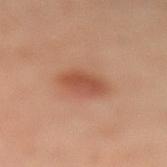<lesion>
<lighting>cross-polarized</lighting>
<lesion_size>
  <long_diameter_mm_approx>4.0</long_diameter_mm_approx>
</lesion_size>
<image>
  <source>total-body photography crop</source>
  <field_of_view_mm>15</field_of_view_mm>
</image>
<site>right leg</site>
<automated_metrics>
  <area_mm2_approx>8.5</area_mm2_approx>
  <shape_asymmetry>0.15</shape_asymmetry>
  <cielab_L>53</cielab_L>
  <cielab_a>26</cielab_a>
  <cielab_b>33</cielab_b>
  <vs_skin_darker_L>10.0</vs_skin_darker_L>
  <nevus_likeness_0_100>100</nevus_likeness_0_100>
  <lesion_detection_confidence_0_100>100</lesion_detection_confidence_0_100>
</automated_metrics>
<patient>
  <sex>female</sex>
  <age_approx>40</age_approx>
</patient>
</lesion>